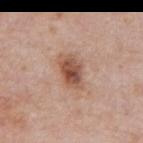| feature | finding |
|---|---|
| workup | imaged on a skin check; not biopsied |
| image source | total-body-photography crop, ~15 mm field of view |
| anatomic site | the mid back |
| automated lesion analysis | a lesion area of about 8 mm², an eccentricity of roughly 0.8, and a symmetry-axis asymmetry near 0.25; a lesion-detection confidence of about 100/100 |
| lesion size | ~4 mm (longest diameter) |
| lighting | white-light |
| subject | male, in their mid-70s |